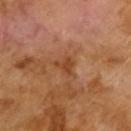Imaged during a routine full-body skin examination; the lesion was not biopsied and no histopathology is available. A 15 mm close-up extracted from a 3D total-body photography capture. A male subject aged 63 to 67.